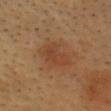biopsy_status: not biopsied; imaged during a skin examination
site: head or neck
lighting: cross-polarized
automated_metrics:
  cielab_L: 42
  cielab_a: 22
  cielab_b: 33
  border_irregularity_0_10: 3.0
  color_variation_0_10: 2.5
  peripheral_color_asymmetry: 1.0
  nevus_likeness_0_100: 25
  lesion_detection_confidence_0_100: 100
patient:
  sex: male
  age_approx: 60
image:
  source: total-body photography crop
  field_of_view_mm: 15